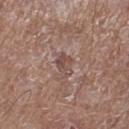The lesion was photographed on a routine skin check and not biopsied; there is no pathology result. The subject is a male about 85 years old or older. The tile uses white-light illumination. Longest diameter approximately 3 mm. Located on the leg. Automated tile analysis of the lesion measured a normalized lesion–skin contrast near 6. A roughly 15 mm field-of-view crop from a total-body skin photograph.